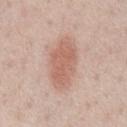Assessment:
Captured during whole-body skin photography for melanoma surveillance; the lesion was not biopsied.
Image and clinical context:
Cropped from a whole-body photographic skin survey; the tile spans about 15 mm. A male subject about 60 years old. The lesion's longest dimension is about 6 mm. Automated image analysis of the tile measured a border-irregularity rating of about 2/10, a within-lesion color-variation index near 2.5/10, and radial color variation of about 1. And it measured a detector confidence of about 100 out of 100 that the crop contains a lesion. On the chest. The tile uses white-light illumination.